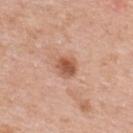Notes:
• notes — imaged on a skin check; not biopsied
• illumination — white-light illumination
• patient — female, aged approximately 40
• acquisition — ~15 mm tile from a whole-body skin photo
• anatomic site — the upper back
• lesion size — ≈2.5 mm